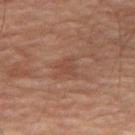This lesion was catalogued during total-body skin photography and was not selected for biopsy.
A male patient, aged 68–72.
The lesion is on the right thigh.
A 15 mm close-up tile from a total-body photography series done for melanoma screening.